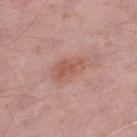Q: Was a biopsy performed?
A: imaged on a skin check; not biopsied
Q: Patient demographics?
A: male, roughly 55 years of age
Q: What is the anatomic site?
A: the left lower leg
Q: How was the tile lit?
A: white-light
Q: How was this image acquired?
A: ~15 mm crop, total-body skin-cancer survey
Q: What did automated image analysis measure?
A: an eccentricity of roughly 0.85 and a symmetry-axis asymmetry near 0.35; a lesion color around L≈55 a*≈24 b*≈28 in CIELAB, about 8 CIELAB-L* units darker than the surrounding skin, and a normalized border contrast of about 6.5; a border-irregularity index near 4/10, a within-lesion color-variation index near 1.5/10, and a peripheral color-asymmetry measure near 0.5
Q: How large is the lesion?
A: ≈3.5 mm